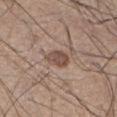notes = catalogued during a skin exam; not biopsied.